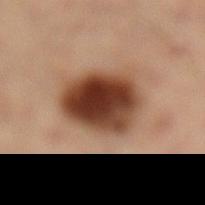| feature | finding |
|---|---|
| notes | no biopsy performed (imaged during a skin exam) |
| patient | male, approximately 50 years of age |
| automated metrics | a mean CIELAB color near L≈41 a*≈22 b*≈30 and roughly 20 lightness units darker than nearby skin; an automated nevus-likeness rating near 100 out of 100 |
| imaging modality | ~15 mm tile from a whole-body skin photo |
| anatomic site | the left leg |
| size | ≈6 mm |